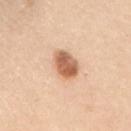| feature | finding |
|---|---|
| workup | total-body-photography surveillance lesion; no biopsy |
| illumination | white-light illumination |
| TBP lesion metrics | a lesion area of about 8.5 mm², an outline eccentricity of about 0.55 (0 = round, 1 = elongated), and two-axis asymmetry of about 0.1; an average lesion color of about L≈62 a*≈22 b*≈35 (CIELAB) and a lesion-to-skin contrast of about 9.5 (normalized; higher = more distinct); border irregularity of about 1 on a 0–10 scale and radial color variation of about 1.5 |
| site | the right upper arm |
| patient | female, aged around 65 |
| image source | ~15 mm tile from a whole-body skin photo |
| diameter | ~3.5 mm (longest diameter) |